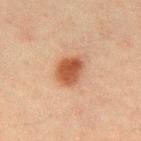Clinical impression:
Recorded during total-body skin imaging; not selected for excision or biopsy.
Context:
Measured at roughly 3.5 mm in maximum diameter. A 15 mm close-up extracted from a 3D total-body photography capture. A male subject aged approximately 50. Located on the mid back.